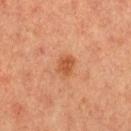From the left thigh. This is a cross-polarized tile. Approximately 3 mm at its widest. The patient is a female roughly 40 years of age. Cropped from a whole-body photographic skin survey; the tile spans about 15 mm.